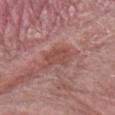This lesion was catalogued during total-body skin photography and was not selected for biopsy. On the left forearm. This image is a 15 mm lesion crop taken from a total-body photograph. A male subject in their mid- to late 70s. An algorithmic analysis of the crop reported a lesion area of about 7.5 mm², a shape eccentricity near 0.6, and a shape-asymmetry score of about 0.35 (0 = symmetric). And it measured roughly 8 lightness units darker than nearby skin and a normalized border contrast of about 6.5. The analysis additionally found internal color variation of about 2.5 on a 0–10 scale and radial color variation of about 1. This is a white-light tile. About 3.5 mm across.